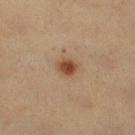Q: Is there a histopathology result?
A: no biopsy performed (imaged during a skin exam)
Q: Lesion location?
A: the right lower leg
Q: Lesion size?
A: about 3 mm
Q: What are the patient's age and sex?
A: female, roughly 40 years of age
Q: What is the imaging modality?
A: ~15 mm tile from a whole-body skin photo
Q: Illumination type?
A: cross-polarized illumination